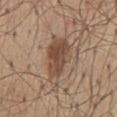site = the mid back
image = ~15 mm crop, total-body skin-cancer survey
TBP lesion metrics = a footprint of about 15 mm² and two-axis asymmetry of about 0.25; a classifier nevus-likeness of about 90/100 and lesion-presence confidence of about 100/100
patient = male, roughly 55 years of age
lesion diameter = ≈7 mm